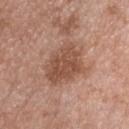Clinical impression:
Imaged during a routine full-body skin examination; the lesion was not biopsied and no histopathology is available.
Background:
Cropped from a whole-body photographic skin survey; the tile spans about 15 mm. A male patient aged approximately 50. Longest diameter approximately 5 mm. The lesion is located on the chest. Captured under white-light illumination. An algorithmic analysis of the crop reported a mean CIELAB color near L≈50 a*≈21 b*≈29, roughly 10 lightness units darker than nearby skin, and a normalized border contrast of about 7.5. The analysis additionally found a border-irregularity index near 3/10 and peripheral color asymmetry of about 1.